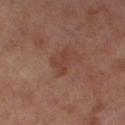Assessment:
The lesion was tiled from a total-body skin photograph and was not biopsied.
Background:
A 15 mm close-up extracted from a 3D total-body photography capture. The lesion-visualizer software estimated a lesion color around L≈34 a*≈19 b*≈23 in CIELAB and a lesion–skin lightness drop of about 5. And it measured radial color variation of about 0. It also reported a classifier nevus-likeness of about 0/100 and lesion-presence confidence of about 100/100. Approximately 2.5 mm at its widest. From the right thigh. A female patient, aged around 60. This is a cross-polarized tile.